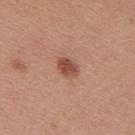This lesion was catalogued during total-body skin photography and was not selected for biopsy. A 15 mm close-up extracted from a 3D total-body photography capture. On the upper back. The lesion-visualizer software estimated a mean CIELAB color near L≈49 a*≈25 b*≈29, about 12 CIELAB-L* units darker than the surrounding skin, and a normalized lesion–skin contrast near 8.5. The analysis additionally found border irregularity of about 1.5 on a 0–10 scale and a within-lesion color-variation index near 2.5/10. And it measured an automated nevus-likeness rating near 95 out of 100 and a lesion-detection confidence of about 100/100. This is a white-light tile. The lesion's longest dimension is about 3 mm. The subject is a female aged approximately 25.